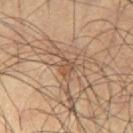Imaged during a routine full-body skin examination; the lesion was not biopsied and no histopathology is available. Imaged with cross-polarized lighting. From the left thigh. A 15 mm close-up extracted from a 3D total-body photography capture. About 3.5 mm across. The patient is a male approximately 60 years of age.